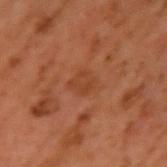This lesion was catalogued during total-body skin photography and was not selected for biopsy.
A male subject aged 58–62.
A lesion tile, about 15 mm wide, cut from a 3D total-body photograph.
From the left upper arm.
This is a cross-polarized tile.
Longest diameter approximately 2.5 mm.